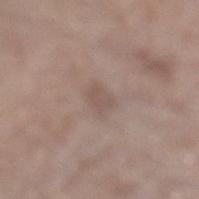biopsy status = imaged on a skin check; not biopsied | illumination = white-light | acquisition = 15 mm crop, total-body photography | location = the left lower leg | size = ≈2.5 mm | patient = male, roughly 65 years of age.